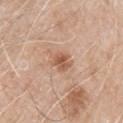Recorded during total-body skin imaging; not selected for excision or biopsy. Automated image analysis of the tile measured a border-irregularity rating of about 2.5/10, a within-lesion color-variation index near 4/10, and radial color variation of about 1.5. The software also gave a classifier nevus-likeness of about 70/100 and a lesion-detection confidence of about 100/100. The tile uses white-light illumination. A male subject, aged 78–82. A lesion tile, about 15 mm wide, cut from a 3D total-body photograph. On the right upper arm. The lesion's longest dimension is about 2.5 mm.